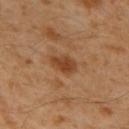<lesion>
<biopsy_status>not biopsied; imaged during a skin examination</biopsy_status>
<lighting>cross-polarized</lighting>
<site>upper back</site>
<patient>
  <sex>male</sex>
  <age_approx>60</age_approx>
</patient>
<image>
  <source>total-body photography crop</source>
  <field_of_view_mm>15</field_of_view_mm>
</image>
<automated_metrics>
  <border_irregularity_0_10>2.0</border_irregularity_0_10>
  <color_variation_0_10>2.5</color_variation_0_10>
  <peripheral_color_asymmetry>1.0</peripheral_color_asymmetry>
  <nevus_likeness_0_100>90</nevus_likeness_0_100>
  <lesion_detection_confidence_0_100>100</lesion_detection_confidence_0_100>
</automated_metrics>
<lesion_size>
  <long_diameter_mm_approx>3.0</long_diameter_mm_approx>
</lesion_size>
</lesion>